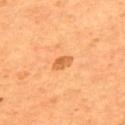<case>
  <lighting>cross-polarized</lighting>
  <patient>
    <sex>male</sex>
    <age_approx>55</age_approx>
  </patient>
  <site>upper back</site>
  <image>
    <source>total-body photography crop</source>
    <field_of_view_mm>15</field_of_view_mm>
  </image>
</case>